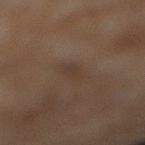Case summary:
• follow-up — catalogued during a skin exam; not biopsied
• lesion size — about 3 mm
• lighting — cross-polarized
• patient — female, roughly 60 years of age
• image source — ~15 mm crop, total-body skin-cancer survey
• location — the right lower leg